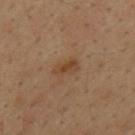notes: no biopsy performed (imaged during a skin exam) | site: the back | automated metrics: an area of roughly 4 mm², an outline eccentricity of about 0.9 (0 = round, 1 = elongated), and two-axis asymmetry of about 0.25; roughly 7 lightness units darker than nearby skin and a lesion-to-skin contrast of about 7 (normalized; higher = more distinct); a border-irregularity rating of about 3/10 and radial color variation of about 1; lesion-presence confidence of about 100/100 | image: total-body-photography crop, ~15 mm field of view | subject: male, about 35 years old | size: ≈3 mm | tile lighting: cross-polarized illumination.